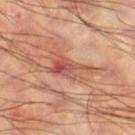The lesion was tiled from a total-body skin photograph and was not biopsied.
The lesion-visualizer software estimated a lesion area of about 10 mm² and an eccentricity of roughly 0.85. The software also gave a nevus-likeness score of about 0/100.
The lesion is on the leg.
A male patient roughly 60 years of age.
A lesion tile, about 15 mm wide, cut from a 3D total-body photograph.
The lesion's longest dimension is about 5.5 mm.
Captured under cross-polarized illumination.